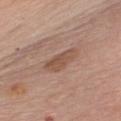Impression:
Part of a total-body skin-imaging series; this lesion was reviewed on a skin check and was not flagged for biopsy.
Context:
A lesion tile, about 15 mm wide, cut from a 3D total-body photograph. The subject is a female aged 63–67. From the chest.